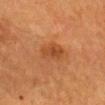Captured during whole-body skin photography for melanoma surveillance; the lesion was not biopsied.
The lesion-visualizer software estimated a classifier nevus-likeness of about 35/100 and a detector confidence of about 100 out of 100 that the crop contains a lesion.
A female patient, aged approximately 55.
The lesion is on the head or neck.
Cropped from a whole-body photographic skin survey; the tile spans about 15 mm.
The tile uses cross-polarized illumination.
The lesion's longest dimension is about 3.5 mm.